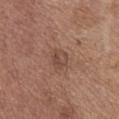This image is a 15 mm lesion crop taken from a total-body photograph. The subject is a female aged 63–67. Automated tile analysis of the lesion measured a lesion color around L≈46 a*≈19 b*≈25 in CIELAB, about 7 CIELAB-L* units darker than the surrounding skin, and a normalized border contrast of about 5.5. The analysis additionally found an automated nevus-likeness rating near 0 out of 100 and lesion-presence confidence of about 100/100. Measured at roughly 2.5 mm in maximum diameter. This is a white-light tile. Located on the chest.